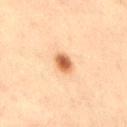<record>
<biopsy_status>not biopsied; imaged during a skin examination</biopsy_status>
<lighting>cross-polarized</lighting>
<image>
  <source>total-body photography crop</source>
  <field_of_view_mm>15</field_of_view_mm>
</image>
<automated_metrics>
  <eccentricity>0.6</eccentricity>
  <shape_asymmetry>0.15</shape_asymmetry>
  <cielab_L>53</cielab_L>
  <cielab_a>22</cielab_a>
  <cielab_b>35</cielab_b>
  <vs_skin_darker_L>15.0</vs_skin_darker_L>
</automated_metrics>
<site>leg</site>
<patient>
  <sex>male</sex>
  <age_approx>70</age_approx>
</patient>
<lesion_size>
  <long_diameter_mm_approx>2.5</long_diameter_mm_approx>
</lesion_size>
</record>